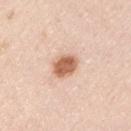<lesion>
  <biopsy_status>not biopsied; imaged during a skin examination</biopsy_status>
</lesion>